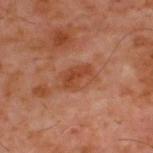Recorded during total-body skin imaging; not selected for excision or biopsy. Imaged with cross-polarized lighting. Approximately 4 mm at its widest. A male patient, about 60 years old. Automated image analysis of the tile measured a mean CIELAB color near L≈41 a*≈26 b*≈32, about 8 CIELAB-L* units darker than the surrounding skin, and a normalized lesion–skin contrast near 7.5. It also reported a nevus-likeness score of about 0/100 and a detector confidence of about 100 out of 100 that the crop contains a lesion. A 15 mm close-up tile from a total-body photography series done for melanoma screening. On the upper back.